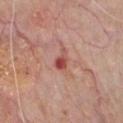Assessment: The lesion was photographed on a routine skin check and not biopsied; there is no pathology result. Background: A 15 mm close-up extracted from a 3D total-body photography capture. A male subject in their 80s. The lesion is located on the chest. About 3 mm across. Imaged with white-light lighting.